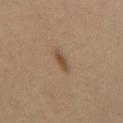biopsy_status: not biopsied; imaged during a skin examination
patient:
  sex: male
  age_approx: 60
image:
  source: total-body photography crop
  field_of_view_mm: 15
lesion_size:
  long_diameter_mm_approx: 2.5
site: mid back
lighting: cross-polarized